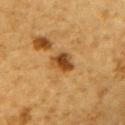Approximately 3 mm at its widest. Imaged with cross-polarized lighting. A 15 mm close-up tile from a total-body photography series done for melanoma screening. From the upper back. A male patient in their mid-80s or older.